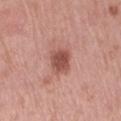Clinical impression:
The lesion was tiled from a total-body skin photograph and was not biopsied.
Clinical summary:
Captured under white-light illumination. Located on the right thigh. A 15 mm crop from a total-body photograph taken for skin-cancer surveillance. A female subject, aged approximately 55.